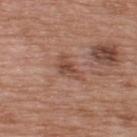biopsy status: catalogued during a skin exam; not biopsied | lighting: white-light illumination | lesion diameter: ≈3.5 mm | subject: male, aged around 50 | site: the upper back | image source: ~15 mm tile from a whole-body skin photo.